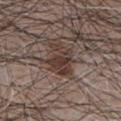Recorded during total-body skin imaging; not selected for excision or biopsy. This is a white-light tile. A region of skin cropped from a whole-body photographic capture, roughly 15 mm wide. A male subject, aged 63–67. Located on the chest.